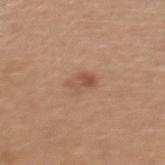Assessment: Part of a total-body skin-imaging series; this lesion was reviewed on a skin check and was not flagged for biopsy. Image and clinical context: The subject is a female aged 53 to 57. The recorded lesion diameter is about 2.5 mm. A 15 mm close-up extracted from a 3D total-body photography capture. Captured under white-light illumination. From the upper back.